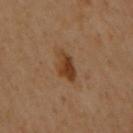Findings:
– notes — total-body-photography surveillance lesion; no biopsy